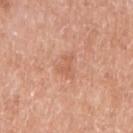Case summary:
• notes: total-body-photography surveillance lesion; no biopsy
• acquisition: ~15 mm crop, total-body skin-cancer survey
• lighting: white-light illumination
• location: the right upper arm
• subject: female, approximately 75 years of age
• lesion diameter: ≈3 mm